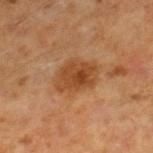subject=male, approximately 60 years of age; lesion size=~4.5 mm (longest diameter); anatomic site=the leg; imaging modality=~15 mm tile from a whole-body skin photo.